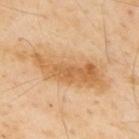workup — catalogued during a skin exam; not biopsied | lesion diameter — about 11 mm | illumination — cross-polarized | acquisition — 15 mm crop, total-body photography | patient — male, in their mid-50s | location — the upper back.